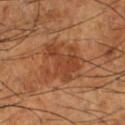Imaged during a routine full-body skin examination; the lesion was not biopsied and no histopathology is available.
The lesion-visualizer software estimated border irregularity of about 7.5 on a 0–10 scale and internal color variation of about 3 on a 0–10 scale. The analysis additionally found a classifier nevus-likeness of about 10/100 and lesion-presence confidence of about 100/100.
Located on the left lower leg.
Imaged with cross-polarized lighting.
A male patient approximately 65 years of age.
A 15 mm close-up tile from a total-body photography series done for melanoma screening.